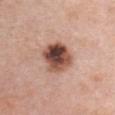follow-up: no biopsy performed (imaged during a skin exam)
image: ~15 mm crop, total-body skin-cancer survey
body site: the chest
size: ≈4 mm
subject: female, roughly 60 years of age
lighting: white-light illumination